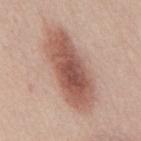Impression:
Captured during whole-body skin photography for melanoma surveillance; the lesion was not biopsied.
Background:
Automated image analysis of the tile measured a mean CIELAB color near L≈56 a*≈21 b*≈26, roughly 14 lightness units darker than nearby skin, and a normalized lesion–skin contrast near 9. The analysis additionally found border irregularity of about 2.5 on a 0–10 scale and a color-variation rating of about 6/10. A male patient about 45 years old. Captured under white-light illumination. A lesion tile, about 15 mm wide, cut from a 3D total-body photograph. The recorded lesion diameter is about 10 mm. The lesion is on the mid back.